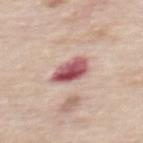Part of a total-body skin-imaging series; this lesion was reviewed on a skin check and was not flagged for biopsy. The tile uses white-light illumination. The lesion is on the back. The lesion-visualizer software estimated a footprint of about 9 mm² and an outline eccentricity of about 0.65 (0 = round, 1 = elongated). The analysis additionally found internal color variation of about 9 on a 0–10 scale and a peripheral color-asymmetry measure near 3. The analysis additionally found an automated nevus-likeness rating near 0 out of 100 and a detector confidence of about 100 out of 100 that the crop contains a lesion. The patient is a male aged around 55. Measured at roughly 4 mm in maximum diameter. Cropped from a total-body skin-imaging series; the visible field is about 15 mm.